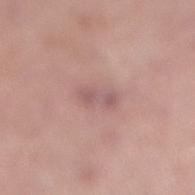biopsy status = catalogued during a skin exam; not biopsied
size = ~3.5 mm (longest diameter)
illumination = white-light
patient = male, aged approximately 50
automated lesion analysis = an average lesion color of about L≈57 a*≈21 b*≈20 (CIELAB), about 8 CIELAB-L* units darker than the surrounding skin, and a normalized lesion–skin contrast near 5.5
image = ~15 mm tile from a whole-body skin photo
site = the right lower leg